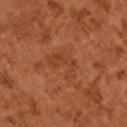Q: Was this lesion biopsied?
A: total-body-photography surveillance lesion; no biopsy
Q: What are the patient's age and sex?
A: male, about 65 years old
Q: What is the imaging modality?
A: ~15 mm crop, total-body skin-cancer survey
Q: What is the lesion's diameter?
A: ≈4 mm
Q: How was the tile lit?
A: cross-polarized
Q: What did automated image analysis measure?
A: a lesion color around L≈40 a*≈26 b*≈36 in CIELAB and a normalized border contrast of about 5; a border-irregularity rating of about 7.5/10 and radial color variation of about 1